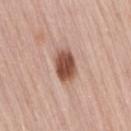Assessment: This lesion was catalogued during total-body skin photography and was not selected for biopsy. Clinical summary: A 15 mm close-up extracted from a 3D total-body photography capture. Captured under white-light illumination. On the right thigh. The subject is a female roughly 65 years of age.